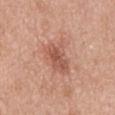Q: Was a biopsy performed?
A: catalogued during a skin exam; not biopsied
Q: Lesion size?
A: ≈5 mm
Q: What did automated image analysis measure?
A: a lesion area of about 12 mm², a shape eccentricity near 0.8, and a shape-asymmetry score of about 0.25 (0 = symmetric); roughly 10 lightness units darker than nearby skin and a lesion-to-skin contrast of about 6.5 (normalized; higher = more distinct); a border-irregularity rating of about 4/10, a color-variation rating of about 3.5/10, and a peripheral color-asymmetry measure near 1; a classifier nevus-likeness of about 30/100 and a lesion-detection confidence of about 100/100
Q: Lesion location?
A: the mid back
Q: What lighting was used for the tile?
A: white-light illumination
Q: What is the imaging modality?
A: ~15 mm crop, total-body skin-cancer survey
Q: Patient demographics?
A: female, about 40 years old